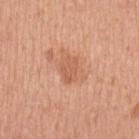Clinical impression: No biopsy was performed on this lesion — it was imaged during a full skin examination and was not determined to be concerning. Clinical summary: The patient is a female aged around 50. A region of skin cropped from a whole-body photographic capture, roughly 15 mm wide. Located on the left upper arm. This is a white-light tile. Approximately 3 mm at its widest.